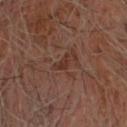Recorded during total-body skin imaging; not selected for excision or biopsy. A 15 mm close-up tile from a total-body photography series done for melanoma screening. Automated tile analysis of the lesion measured a lesion color around L≈31 a*≈21 b*≈25 in CIELAB, a lesion–skin lightness drop of about 7, and a normalized lesion–skin contrast near 7. This is a cross-polarized tile. A male subject in their mid-60s.